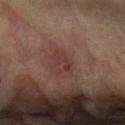Part of a total-body skin-imaging series; this lesion was reviewed on a skin check and was not flagged for biopsy. A 15 mm crop from a total-body photograph taken for skin-cancer surveillance. The lesion's longest dimension is about 3.5 mm. This is a cross-polarized tile. A female patient about 75 years old. The lesion is on the left forearm.